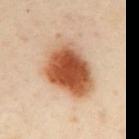Assessment:
This lesion was catalogued during total-body skin photography and was not selected for biopsy.
Context:
A female patient approximately 40 years of age. A lesion tile, about 15 mm wide, cut from a 3D total-body photograph. The lesion is located on the upper back. Automated image analysis of the tile measured an outline eccentricity of about 0.7 (0 = round, 1 = elongated) and a shape-asymmetry score of about 0.25 (0 = symmetric). The analysis additionally found a nevus-likeness score of about 100/100. About 6.5 mm across.